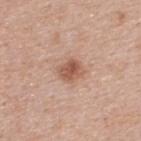Captured during whole-body skin photography for melanoma surveillance; the lesion was not biopsied. Imaged with white-light lighting. Longest diameter approximately 2.5 mm. A roughly 15 mm field-of-view crop from a total-body skin photograph. A male patient, in their 40s. From the upper back.